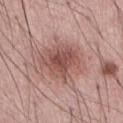A male subject approximately 55 years of age. A region of skin cropped from a whole-body photographic capture, roughly 15 mm wide. The lesion is on the abdomen.